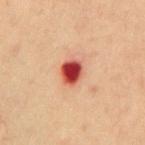<record>
  <biopsy_status>not biopsied; imaged during a skin examination</biopsy_status>
  <patient>
    <sex>male</sex>
    <age_approx>70</age_approx>
  </patient>
  <site>abdomen</site>
  <lesion_size>
    <long_diameter_mm_approx>3.0</long_diameter_mm_approx>
  </lesion_size>
  <image>
    <source>total-body photography crop</source>
    <field_of_view_mm>15</field_of_view_mm>
  </image>
  <automated_metrics>
    <cielab_L>47</cielab_L>
    <cielab_a>38</cielab_a>
    <cielab_b>32</cielab_b>
    <vs_skin_darker_L>22.0</vs_skin_darker_L>
    <vs_skin_contrast_norm>14.0</vs_skin_contrast_norm>
  </automated_metrics>
</record>